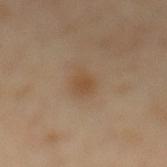The lesion was photographed on a routine skin check and not biopsied; there is no pathology result.
Approximately 3 mm at its widest.
A close-up tile cropped from a whole-body skin photograph, about 15 mm across.
The patient is a male about 65 years old.
On the back.